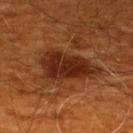No biopsy was performed on this lesion — it was imaged during a full skin examination and was not determined to be concerning. A male patient, in their 60s. A 15 mm close-up extracted from a 3D total-body photography capture. Captured under cross-polarized illumination. The lesion's longest dimension is about 7 mm. From the upper back.